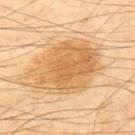The lesion was tiled from a total-body skin photograph and was not biopsied.
The patient is a male about 60 years old.
Captured under cross-polarized illumination.
This image is a 15 mm lesion crop taken from a total-body photograph.
An algorithmic analysis of the crop reported a lesion area of about 36 mm², an outline eccentricity of about 0.7 (0 = round, 1 = elongated), and a shape-asymmetry score of about 0.15 (0 = symmetric). And it measured a mean CIELAB color near L≈65 a*≈20 b*≈44, about 11 CIELAB-L* units darker than the surrounding skin, and a normalized lesion–skin contrast near 7.5. It also reported a color-variation rating of about 5/10 and radial color variation of about 1.5.
Located on the back.